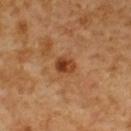  biopsy_status: not biopsied; imaged during a skin examination
  automated_metrics:
    area_mm2_approx: 4.5
    eccentricity: 0.55
    shape_asymmetry: 0.2
    border_irregularity_0_10: 1.5
    color_variation_0_10: 6.5
    peripheral_color_asymmetry: 2.5
  lighting: cross-polarized
  image:
    source: total-body photography crop
    field_of_view_mm: 15
  site: back
  lesion_size:
    long_diameter_mm_approx: 2.5
  patient:
    sex: male
    age_approx: 60